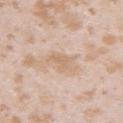notes: imaged on a skin check; not biopsied
image-analysis metrics: a lesion color around L≈67 a*≈16 b*≈31 in CIELAB, a lesion–skin lightness drop of about 7, and a normalized lesion–skin contrast near 5.5
subject: female, about 25 years old
acquisition: ~15 mm crop, total-body skin-cancer survey
illumination: white-light
body site: the left upper arm
size: ≈3 mm